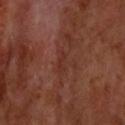Captured during whole-body skin photography for melanoma surveillance; the lesion was not biopsied. Cropped from a total-body skin-imaging series; the visible field is about 15 mm. A male patient aged 68–72. Automated image analysis of the tile measured an area of roughly 2.5 mm², an outline eccentricity of about 0.95 (0 = round, 1 = elongated), and a symmetry-axis asymmetry near 0.35. The software also gave an automated nevus-likeness rating near 0 out of 100 and a detector confidence of about 80 out of 100 that the crop contains a lesion. The lesion is on the head or neck.